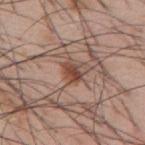<record>
<lighting>white-light</lighting>
<site>mid back</site>
<patient>
  <sex>male</sex>
  <age_approx>55</age_approx>
</patient>
<image>
  <source>total-body photography crop</source>
  <field_of_view_mm>15</field_of_view_mm>
</image>
<lesion_size>
  <long_diameter_mm_approx>3.0</long_diameter_mm_approx>
</lesion_size>
</record>